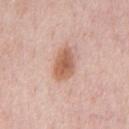This lesion was catalogued during total-body skin photography and was not selected for biopsy.
On the chest.
A 15 mm crop from a total-body photograph taken for skin-cancer surveillance.
The patient is a male aged 48–52.
Imaged with white-light lighting.
Measured at roughly 4 mm in maximum diameter.